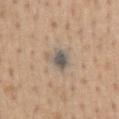| field | value |
|---|---|
| body site | the front of the torso |
| subject | male, about 65 years old |
| image | 15 mm crop, total-body photography |